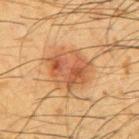No biopsy was performed on this lesion — it was imaged during a full skin examination and was not determined to be concerning. The subject is a male roughly 35 years of age. This is a cross-polarized tile. Cropped from a total-body skin-imaging series; the visible field is about 15 mm. On the right upper arm. The recorded lesion diameter is about 5.5 mm.